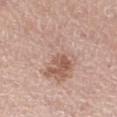| key | value |
|---|---|
| workup | no biopsy performed (imaged during a skin exam) |
| subject | female, about 65 years old |
| tile lighting | white-light |
| imaging modality | total-body-photography crop, ~15 mm field of view |
| site | the left thigh |
| automated metrics | an outline eccentricity of about 0.9 (0 = round, 1 = elongated) and a symmetry-axis asymmetry near 0.4; a mean CIELAB color near L≈61 a*≈18 b*≈26, roughly 8 lightness units darker than nearby skin, and a lesion-to-skin contrast of about 5 (normalized; higher = more distinct); border irregularity of about 5.5 on a 0–10 scale, a color-variation rating of about 8.5/10, and a peripheral color-asymmetry measure near 3; an automated nevus-likeness rating near 5 out of 100 and a lesion-detection confidence of about 100/100 |